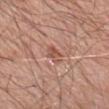{"biopsy_status": "not biopsied; imaged during a skin examination", "patient": {"sex": "male", "age_approx": 80}, "lesion_size": {"long_diameter_mm_approx": 3.5}, "site": "back", "lighting": "white-light", "image": {"source": "total-body photography crop", "field_of_view_mm": 15}}